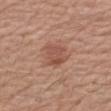  biopsy_status: not biopsied; imaged during a skin examination